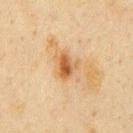This lesion was catalogued during total-body skin photography and was not selected for biopsy. From the front of the torso. A roughly 15 mm field-of-view crop from a total-body skin photograph. A male subject, aged 58 to 62.